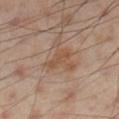Case summary:
* workup — imaged on a skin check; not biopsied
* site — the right thigh
* subject — male, in their mid- to late 50s
* lighting — cross-polarized illumination
* TBP lesion metrics — a footprint of about 3 mm², a shape eccentricity near 0.95, and a shape-asymmetry score of about 0.25 (0 = symmetric)
* size — ~3 mm (longest diameter)
* image source — total-body-photography crop, ~15 mm field of view